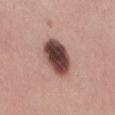Recorded during total-body skin imaging; not selected for excision or biopsy.
A 15 mm close-up extracted from a 3D total-body photography capture.
Longest diameter approximately 5 mm.
From the back.
Imaged with white-light lighting.
The patient is a female approximately 30 years of age.
Automated image analysis of the tile measured a border-irregularity index near 1.5/10 and peripheral color asymmetry of about 2.